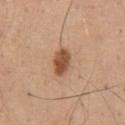Impression: The lesion was tiled from a total-body skin photograph and was not biopsied. Clinical summary: Measured at roughly 3.5 mm in maximum diameter. Imaged with white-light lighting. A 15 mm close-up extracted from a 3D total-body photography capture. A male subject aged 43–47. On the mid back. The total-body-photography lesion software estimated roughly 14 lightness units darker than nearby skin and a lesion-to-skin contrast of about 9.5 (normalized; higher = more distinct). The analysis additionally found a border-irregularity rating of about 2/10 and internal color variation of about 3.5 on a 0–10 scale. It also reported a nevus-likeness score of about 95/100 and lesion-presence confidence of about 100/100.